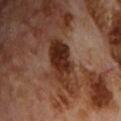  biopsy_status: not biopsied; imaged during a skin examination
  site: front of the torso
  image:
    source: total-body photography crop
    field_of_view_mm: 15
  lesion_size:
    long_diameter_mm_approx: 6.0
  lighting: cross-polarized
  patient:
    sex: male
    age_approx: 70
  automated_metrics:
    area_mm2_approx: 12.0
    eccentricity: 0.9
    border_irregularity_0_10: 4.0
    color_variation_0_10: 4.5
    peripheral_color_asymmetry: 1.5
    nevus_likeness_0_100: 85
    lesion_detection_confidence_0_100: 100